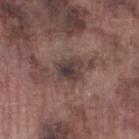Clinical impression:
The lesion was photographed on a routine skin check and not biopsied; there is no pathology result.
Acquisition and patient details:
A lesion tile, about 15 mm wide, cut from a 3D total-body photograph. This is a white-light tile. A male patient aged 73–77. The lesion is on the left thigh. The recorded lesion diameter is about 3.5 mm. Automated image analysis of the tile measured an average lesion color of about L≈38 a*≈15 b*≈16 (CIELAB) and a lesion–skin lightness drop of about 10. The software also gave a border-irregularity index near 3/10, a within-lesion color-variation index near 5.5/10, and peripheral color asymmetry of about 1.5.